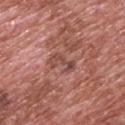Clinical impression:
The lesion was photographed on a routine skin check and not biopsied; there is no pathology result.
Context:
The tile uses white-light illumination. A male subject, aged 68 to 72. A region of skin cropped from a whole-body photographic capture, roughly 15 mm wide. The lesion is on the upper back.